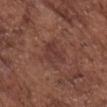– biopsy status — imaged on a skin check; not biopsied
– imaging modality — 15 mm crop, total-body photography
– image-analysis metrics — a lesion color around L≈36 a*≈22 b*≈23 in CIELAB, roughly 6 lightness units darker than nearby skin, and a normalized lesion–skin contrast near 6; a nevus-likeness score of about 0/100 and lesion-presence confidence of about 100/100
– tile lighting — white-light illumination
– subject — male, about 75 years old
– diameter — ≈3.5 mm
– anatomic site — the chest